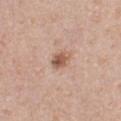The lesion was photographed on a routine skin check and not biopsied; there is no pathology result. The lesion is on the chest. The tile uses white-light illumination. The patient is a male about 40 years old. The recorded lesion diameter is about 3 mm. A 15 mm close-up extracted from a 3D total-body photography capture.